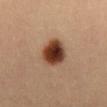Assessment:
Imaged during a routine full-body skin examination; the lesion was not biopsied and no histopathology is available.
Background:
A male patient approximately 20 years of age. Captured under cross-polarized illumination. A roughly 15 mm field-of-view crop from a total-body skin photograph. From the chest.